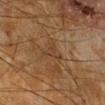The lesion was tiled from a total-body skin photograph and was not biopsied.
A lesion tile, about 15 mm wide, cut from a 3D total-body photograph.
The lesion's longest dimension is about 3 mm.
Imaged with cross-polarized lighting.
The patient is a male about 65 years old.
On the leg.